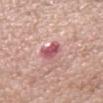Q: Is there a histopathology result?
A: no biopsy performed (imaged during a skin exam)
Q: How large is the lesion?
A: ≈3.5 mm
Q: Patient demographics?
A: female, roughly 55 years of age
Q: How was the tile lit?
A: white-light illumination
Q: What is the imaging modality?
A: total-body-photography crop, ~15 mm field of view
Q: What is the anatomic site?
A: the right lower leg